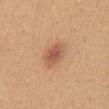- imaging modality — 15 mm crop, total-body photography
- lesion size — ~3 mm (longest diameter)
- location — the abdomen
- patient — female, roughly 25 years of age